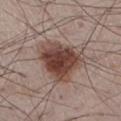  biopsy_status: not biopsied; imaged during a skin examination
  lesion_size:
    long_diameter_mm_approx: 6.0
  site: left thigh
  patient:
    sex: male
    age_approx: 75
  image:
    source: total-body photography crop
    field_of_view_mm: 15
  lighting: white-light